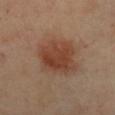notes: imaged on a skin check; not biopsied | anatomic site: the chest | automated metrics: a lesion-to-skin contrast of about 8 (normalized; higher = more distinct); peripheral color asymmetry of about 1; a detector confidence of about 100 out of 100 that the crop contains a lesion | acquisition: 15 mm crop, total-body photography | patient: female, roughly 40 years of age | lighting: cross-polarized illumination.